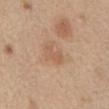{
  "biopsy_status": "not biopsied; imaged during a skin examination",
  "patient": {
    "sex": "male",
    "age_approx": 70
  },
  "lighting": "white-light",
  "automated_metrics": {
    "cielab_L": 58,
    "cielab_a": 20,
    "cielab_b": 32,
    "vs_skin_contrast_norm": 4.5,
    "border_irregularity_0_10": 5.0,
    "color_variation_0_10": 0.5,
    "peripheral_color_asymmetry": 0.0
  },
  "site": "abdomen",
  "image": {
    "source": "total-body photography crop",
    "field_of_view_mm": 15
  }
}